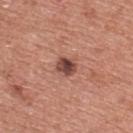Impression: The lesion was tiled from a total-body skin photograph and was not biopsied. Clinical summary: Captured under white-light illumination. A region of skin cropped from a whole-body photographic capture, roughly 15 mm wide. From the chest. Automated image analysis of the tile measured a mean CIELAB color near L≈46 a*≈23 b*≈25, about 15 CIELAB-L* units darker than the surrounding skin, and a lesion-to-skin contrast of about 10.5 (normalized; higher = more distinct). It also reported a border-irregularity index near 1.5/10, internal color variation of about 6 on a 0–10 scale, and peripheral color asymmetry of about 2. And it measured a nevus-likeness score of about 45/100 and a lesion-detection confidence of about 100/100. A male subject approximately 45 years of age.